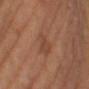workup — imaged on a skin check; not biopsied.